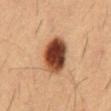illumination: cross-polarized illumination | patient: male, in their mid-50s | anatomic site: the chest | size: ~5.5 mm (longest diameter) | imaging modality: 15 mm crop, total-body photography.